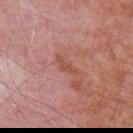<tbp_lesion>
<biopsy_status>not biopsied; imaged during a skin examination</biopsy_status>
<lesion_size>
  <long_diameter_mm_approx>3.0</long_diameter_mm_approx>
</lesion_size>
<lighting>white-light</lighting>
<site>right upper arm</site>
<image>
  <source>total-body photography crop</source>
  <field_of_view_mm>15</field_of_view_mm>
</image>
<automated_metrics>
  <nevus_likeness_0_100>0</nevus_likeness_0_100>
  <lesion_detection_confidence_0_100>95</lesion_detection_confidence_0_100>
</automated_metrics>
<patient>
  <sex>male</sex>
  <age_approx>80</age_approx>
</patient>
</tbp_lesion>